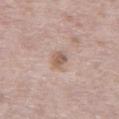Q: Is there a histopathology result?
A: catalogued during a skin exam; not biopsied
Q: What lighting was used for the tile?
A: white-light
Q: What did automated image analysis measure?
A: a lesion area of about 3.5 mm², a shape eccentricity near 0.8, and a shape-asymmetry score of about 0.2 (0 = symmetric); roughly 11 lightness units darker than nearby skin and a lesion-to-skin contrast of about 7.5 (normalized; higher = more distinct); a nevus-likeness score of about 15/100
Q: Lesion size?
A: ~2.5 mm (longest diameter)
Q: How was this image acquired?
A: ~15 mm crop, total-body skin-cancer survey
Q: Where on the body is the lesion?
A: the chest
Q: Patient demographics?
A: male, in their mid-60s